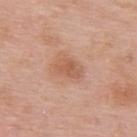Q: Is there a histopathology result?
A: catalogued during a skin exam; not biopsied
Q: Lesion location?
A: the back
Q: Lesion size?
A: about 3.5 mm
Q: How was this image acquired?
A: ~15 mm tile from a whole-body skin photo
Q: Who is the patient?
A: female, approximately 50 years of age